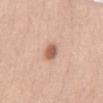Part of a total-body skin-imaging series; this lesion was reviewed on a skin check and was not flagged for biopsy.